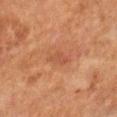{"biopsy_status": "not biopsied; imaged during a skin examination", "site": "leg", "image": {"source": "total-body photography crop", "field_of_view_mm": 15}, "patient": {"sex": "male", "age_approx": 65}}